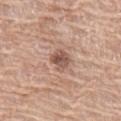Q: Is there a histopathology result?
A: total-body-photography surveillance lesion; no biopsy
Q: What is the imaging modality?
A: 15 mm crop, total-body photography
Q: Who is the patient?
A: female, roughly 60 years of age
Q: What lighting was used for the tile?
A: white-light illumination
Q: Lesion location?
A: the left thigh